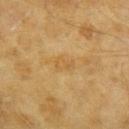– notes — no biopsy performed (imaged during a skin exam)
– image-analysis metrics — a lesion area of about 3 mm², an outline eccentricity of about 0.9 (0 = round, 1 = elongated), and a symmetry-axis asymmetry near 0.35; a classifier nevus-likeness of about 0/100 and a lesion-detection confidence of about 100/100
– acquisition — total-body-photography crop, ~15 mm field of view
– tile lighting — cross-polarized illumination
– site — the right upper arm
– diameter — about 2.5 mm
– patient — female, aged 58–62